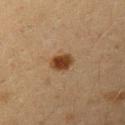Clinical impression: The lesion was photographed on a routine skin check and not biopsied; there is no pathology result. Clinical summary: This image is a 15 mm lesion crop taken from a total-body photograph. Imaged with cross-polarized lighting. A female patient aged approximately 40. The lesion is located on the arm. Automated image analysis of the tile measured a nevus-likeness score of about 100/100 and a detector confidence of about 100 out of 100 that the crop contains a lesion.